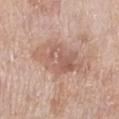<lesion>
  <biopsy_status>not biopsied; imaged during a skin examination</biopsy_status>
  <lighting>white-light</lighting>
  <patient>
    <sex>female</sex>
    <age_approx>70</age_approx>
  </patient>
  <lesion_size>
    <long_diameter_mm_approx>7.5</long_diameter_mm_approx>
  </lesion_size>
  <automated_metrics>
    <area_mm2_approx>20.0</area_mm2_approx>
    <shape_asymmetry>0.25</shape_asymmetry>
    <cielab_L>59</cielab_L>
    <cielab_a>20</cielab_a>
    <cielab_b>27</cielab_b>
    <vs_skin_contrast_norm>6.0</vs_skin_contrast_norm>
    <border_irregularity_0_10>3.5</border_irregularity_0_10>
    <color_variation_0_10>5.5</color_variation_0_10>
    <peripheral_color_asymmetry>2.0</peripheral_color_asymmetry>
  </automated_metrics>
  <image>
    <source>total-body photography crop</source>
    <field_of_view_mm>15</field_of_view_mm>
  </image>
  <site>leg</site>
</lesion>